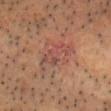{"biopsy_status": "not biopsied; imaged during a skin examination", "site": "head or neck", "lesion_size": {"long_diameter_mm_approx": 5.5}, "lighting": "cross-polarized", "automated_metrics": {"cielab_L": 50, "cielab_a": 23, "cielab_b": 27, "vs_skin_contrast_norm": 5.5, "border_irregularity_0_10": 5.0, "peripheral_color_asymmetry": 1.5}, "patient": {"sex": "male", "age_approx": 65}, "image": {"source": "total-body photography crop", "field_of_view_mm": 15}}